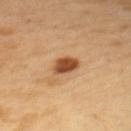Imaged with cross-polarized lighting. Located on the upper back. A lesion tile, about 15 mm wide, cut from a 3D total-body photograph. The lesion's longest dimension is about 3.5 mm. A male patient, aged around 50.